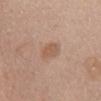{
  "biopsy_status": "not biopsied; imaged during a skin examination",
  "automated_metrics": {
    "border_irregularity_0_10": 2.0,
    "color_variation_0_10": 1.5,
    "peripheral_color_asymmetry": 0.5
  },
  "lighting": "white-light",
  "site": "abdomen",
  "lesion_size": {
    "long_diameter_mm_approx": 2.5
  },
  "image": {
    "source": "total-body photography crop",
    "field_of_view_mm": 15
  },
  "patient": {
    "sex": "female",
    "age_approx": 60
  }
}